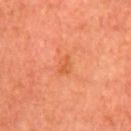Findings:
- workup — total-body-photography surveillance lesion; no biopsy
- lesion diameter — ≈2.5 mm
- image — ~15 mm crop, total-body skin-cancer survey
- patient — male, aged around 65
- body site — the chest
- illumination — cross-polarized